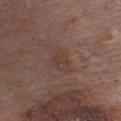Recorded during total-body skin imaging; not selected for excision or biopsy.
A roughly 15 mm field-of-view crop from a total-body skin photograph.
The lesion is on the front of the torso.
The patient is a male aged 78 to 82.
The lesion-visualizer software estimated a footprint of about 6 mm², an outline eccentricity of about 0.8 (0 = round, 1 = elongated), and a symmetry-axis asymmetry near 0.3. And it measured a mean CIELAB color near L≈39 a*≈18 b*≈23 and roughly 5 lightness units darker than nearby skin.